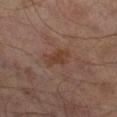The lesion was photographed on a routine skin check and not biopsied; there is no pathology result. From the leg. An algorithmic analysis of the crop reported an eccentricity of roughly 0.85 and a symmetry-axis asymmetry near 0.25. And it measured a lesion color around L≈36 a*≈19 b*≈26 in CIELAB and about 6 CIELAB-L* units darker than the surrounding skin. The software also gave a border-irregularity rating of about 2.5/10, internal color variation of about 1 on a 0–10 scale, and peripheral color asymmetry of about 0.5. The analysis additionally found a classifier nevus-likeness of about 0/100 and a lesion-detection confidence of about 100/100. A male subject aged approximately 65. Longest diameter approximately 3 mm. The tile uses cross-polarized illumination. A close-up tile cropped from a whole-body skin photograph, about 15 mm across.